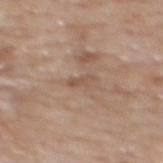| feature | finding |
|---|---|
| notes | imaged on a skin check; not biopsied |
| anatomic site | the upper back |
| subject | female, aged 58 to 62 |
| TBP lesion metrics | an eccentricity of roughly 0.95 and a shape-asymmetry score of about 0.3 (0 = symmetric); a lesion color around L≈53 a*≈17 b*≈27 in CIELAB, about 7 CIELAB-L* units darker than the surrounding skin, and a normalized border contrast of about 5.5; a border-irregularity index near 3.5/10, internal color variation of about 0 on a 0–10 scale, and peripheral color asymmetry of about 0; lesion-presence confidence of about 55/100 |
| acquisition | ~15 mm tile from a whole-body skin photo |
| size | ≈3 mm |